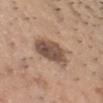workup=total-body-photography surveillance lesion; no biopsy | patient=male, in their mid- to late 30s | site=the head or neck | image=total-body-photography crop, ~15 mm field of view | lesion diameter=about 5 mm.